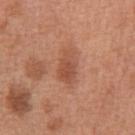No biopsy was performed on this lesion — it was imaged during a full skin examination and was not determined to be concerning.
A roughly 15 mm field-of-view crop from a total-body skin photograph.
The patient is a male in their mid- to late 60s.
On the abdomen.
The total-body-photography lesion software estimated a lesion color around L≈52 a*≈25 b*≈32 in CIELAB.
Approximately 4 mm at its widest.
Captured under white-light illumination.